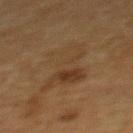Recorded during total-body skin imaging; not selected for excision or biopsy.
From the upper back.
A 15 mm close-up extracted from a 3D total-body photography capture.
A female patient aged 53 to 57.
About 6 mm across.
The lesion-visualizer software estimated a lesion area of about 20 mm², a shape eccentricity near 0.7, and two-axis asymmetry of about 0.25. The software also gave an automated nevus-likeness rating near 0 out of 100 and a detector confidence of about 100 out of 100 that the crop contains a lesion.
The tile uses cross-polarized illumination.